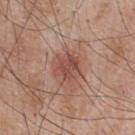Captured during whole-body skin photography for melanoma surveillance; the lesion was not biopsied.
This image is a 15 mm lesion crop taken from a total-body photograph.
From the upper back.
The recorded lesion diameter is about 3 mm.
A male patient in their mid- to late 60s.